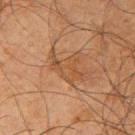This lesion was catalogued during total-body skin photography and was not selected for biopsy. The tile uses cross-polarized illumination. A male patient aged approximately 65. The lesion is on the right upper arm. A region of skin cropped from a whole-body photographic capture, roughly 15 mm wide. The lesion-visualizer software estimated an average lesion color of about L≈41 a*≈18 b*≈31 (CIELAB), roughly 6 lightness units darker than nearby skin, and a normalized lesion–skin contrast near 5.5. The analysis additionally found a border-irregularity rating of about 7/10. Measured at roughly 5 mm in maximum diameter.